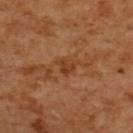Clinical impression: The lesion was tiled from a total-body skin photograph and was not biopsied. Background: On the upper back. The patient is a female aged around 55. Captured under cross-polarized illumination. A close-up tile cropped from a whole-body skin photograph, about 15 mm across. The lesion's longest dimension is about 2.5 mm.